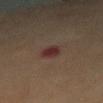No biopsy was performed on this lesion — it was imaged during a full skin examination and was not determined to be concerning.
The recorded lesion diameter is about 2.5 mm.
Located on the mid back.
The tile uses cross-polarized illumination.
Automated tile analysis of the lesion measured an average lesion color of about L≈25 a*≈19 b*≈17 (CIELAB), about 9 CIELAB-L* units darker than the surrounding skin, and a lesion-to-skin contrast of about 10 (normalized; higher = more distinct). The software also gave a border-irregularity index near 2/10, internal color variation of about 2 on a 0–10 scale, and a peripheral color-asymmetry measure near 0.5.
A lesion tile, about 15 mm wide, cut from a 3D total-body photograph.
A female patient aged 53–57.